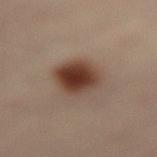| key | value |
|---|---|
| biopsy status | no biopsy performed (imaged during a skin exam) |
| illumination | cross-polarized |
| anatomic site | the lower back |
| lesion size | ~5 mm (longest diameter) |
| TBP lesion metrics | a shape eccentricity near 0.65 and two-axis asymmetry of about 0.1; a mean CIELAB color near L≈41 a*≈18 b*≈26 and a lesion–skin lightness drop of about 14; border irregularity of about 1.5 on a 0–10 scale, a within-lesion color-variation index near 7/10, and a peripheral color-asymmetry measure near 1.5; a classifier nevus-likeness of about 100/100 and lesion-presence confidence of about 100/100 |
| patient | female, aged 38 to 42 |
| imaging modality | ~15 mm tile from a whole-body skin photo |